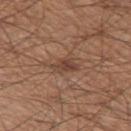No biopsy was performed on this lesion — it was imaged during a full skin examination and was not determined to be concerning. The recorded lesion diameter is about 3.5 mm. The lesion is located on the left thigh. A male patient, in their mid- to late 60s. Captured under white-light illumination. This image is a 15 mm lesion crop taken from a total-body photograph.